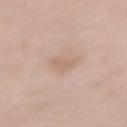Part of a total-body skin-imaging series; this lesion was reviewed on a skin check and was not flagged for biopsy.
The tile uses white-light illumination.
The lesion-visualizer software estimated a footprint of about 5 mm². The analysis additionally found a mean CIELAB color near L≈64 a*≈17 b*≈28 and about 6 CIELAB-L* units darker than the surrounding skin.
A 15 mm close-up tile from a total-body photography series done for melanoma screening.
On the front of the torso.
The subject is a female roughly 65 years of age.